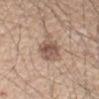Part of a total-body skin-imaging series; this lesion was reviewed on a skin check and was not flagged for biopsy. Cropped from a whole-body photographic skin survey; the tile spans about 15 mm. The lesion is on the arm. A male subject in their 60s.